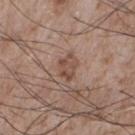A male patient, roughly 75 years of age.
This image is a 15 mm lesion crop taken from a total-body photograph.
Imaged with white-light lighting.
About 3.5 mm across.
From the chest.
An algorithmic analysis of the crop reported a lesion area of about 6 mm². The analysis additionally found lesion-presence confidence of about 100/100.